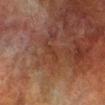{"biopsy_status": "not biopsied; imaged during a skin examination", "lesion_size": {"long_diameter_mm_approx": 2.5}, "patient": {"sex": "male", "age_approx": 70}, "image": {"source": "total-body photography crop", "field_of_view_mm": 15}, "automated_metrics": {"color_variation_0_10": 0.0, "peripheral_color_asymmetry": 0.0, "nevus_likeness_0_100": 0, "lesion_detection_confidence_0_100": 100}, "lighting": "cross-polarized", "site": "right lower leg"}